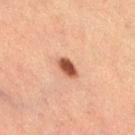Captured during whole-body skin photography for melanoma surveillance; the lesion was not biopsied.
A close-up tile cropped from a whole-body skin photograph, about 15 mm across.
The total-body-photography lesion software estimated an average lesion color of about L≈43 a*≈22 b*≈29 (CIELAB). The software also gave border irregularity of about 1.5 on a 0–10 scale and internal color variation of about 2 on a 0–10 scale. It also reported a nevus-likeness score of about 100/100 and a lesion-detection confidence of about 100/100.
A female subject, in their 60s.
The lesion is located on the right thigh.